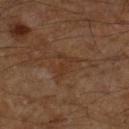Impression:
The lesion was photographed on a routine skin check and not biopsied; there is no pathology result.
Clinical summary:
The lesion is located on the right lower leg. Captured under cross-polarized illumination. A 15 mm close-up extracted from a 3D total-body photography capture. A male subject about 60 years old. The lesion's longest dimension is about 3 mm.